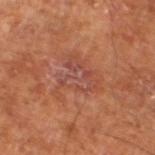{"patient": {"sex": "male", "age_approx": 60}, "site": "right leg", "image": {"source": "total-body photography crop", "field_of_view_mm": 15}}